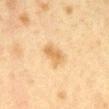| key | value |
|---|---|
| workup | total-body-photography surveillance lesion; no biopsy |
| site | the chest |
| TBP lesion metrics | an area of roughly 5.5 mm², an eccentricity of roughly 0.75, and a symmetry-axis asymmetry near 0.2; a mean CIELAB color near L≈58 a*≈15 b*≈37; a nevus-likeness score of about 60/100 and a detector confidence of about 100 out of 100 that the crop contains a lesion |
| lesion size | about 3 mm |
| tile lighting | cross-polarized |
| image source | ~15 mm crop, total-body skin-cancer survey |
| patient | female, aged around 40 |